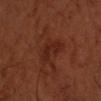Clinical impression: The lesion was photographed on a routine skin check and not biopsied; there is no pathology result. Context: From the left forearm. Cropped from a whole-body photographic skin survey; the tile spans about 15 mm. A male patient, in their 50s.